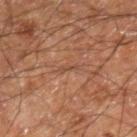notes — total-body-photography surveillance lesion; no biopsy | subject — male, aged 58 to 62 | site — the right lower leg | image source — 15 mm crop, total-body photography.